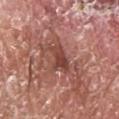Background:
Cropped from a total-body skin-imaging series; the visible field is about 15 mm. The subject is a male aged 63 to 67. On the head or neck.